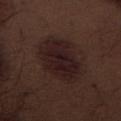A lesion tile, about 15 mm wide, cut from a 3D total-body photograph.
A male subject, aged 68–72.
Automated image analysis of the tile measured a mean CIELAB color near L≈18 a*≈16 b*≈15, roughly 7 lightness units darker than nearby skin, and a normalized border contrast of about 10. The software also gave a detector confidence of about 100 out of 100 that the crop contains a lesion.
About 6.5 mm across.
Located on the abdomen.